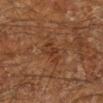Assessment:
The lesion was tiled from a total-body skin photograph and was not biopsied.
Background:
Located on the left lower leg. A male subject, aged 58 to 62. Captured under cross-polarized illumination. This image is a 15 mm lesion crop taken from a total-body photograph.